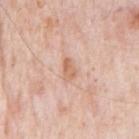This lesion was catalogued during total-body skin photography and was not selected for biopsy.
This is a white-light tile.
Cropped from a whole-body photographic skin survey; the tile spans about 15 mm.
The recorded lesion diameter is about 2.5 mm.
Automated tile analysis of the lesion measured a lesion color around L≈65 a*≈21 b*≈32 in CIELAB, a lesion–skin lightness drop of about 9, and a normalized border contrast of about 7. The analysis additionally found a border-irregularity index near 2.5/10, internal color variation of about 1 on a 0–10 scale, and a peripheral color-asymmetry measure near 0.5. The software also gave a classifier nevus-likeness of about 0/100 and lesion-presence confidence of about 100/100.
The lesion is located on the chest.
A male subject, about 80 years old.